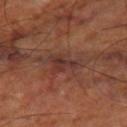Findings:
– biopsy status: total-body-photography surveillance lesion; no biopsy
– lighting: cross-polarized illumination
– automated lesion analysis: a lesion area of about 8 mm² and two-axis asymmetry of about 0.3
– subject: aged 63 to 67
– anatomic site: the right thigh
– image: ~15 mm crop, total-body skin-cancer survey
– lesion size: about 4.5 mm